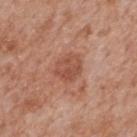{"biopsy_status": "not biopsied; imaged during a skin examination", "lighting": "white-light", "image": {"source": "total-body photography crop", "field_of_view_mm": 15}, "lesion_size": {"long_diameter_mm_approx": 3.5}, "site": "back", "patient": {"sex": "male", "age_approx": 65}}